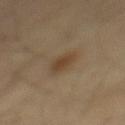Clinical impression: Captured during whole-body skin photography for melanoma surveillance; the lesion was not biopsied. Context: Cropped from a whole-body photographic skin survey; the tile spans about 15 mm. From the mid back. The subject is a male aged around 40.